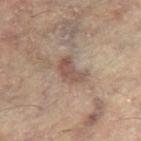Q: Was this lesion biopsied?
A: imaged on a skin check; not biopsied
Q: What is the lesion's diameter?
A: ~3.5 mm (longest diameter)
Q: Patient demographics?
A: female, approximately 80 years of age
Q: How was this image acquired?
A: ~15 mm crop, total-body skin-cancer survey
Q: Where on the body is the lesion?
A: the leg